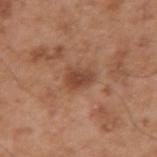Clinical impression: Part of a total-body skin-imaging series; this lesion was reviewed on a skin check and was not flagged for biopsy. Acquisition and patient details: A male patient in their mid- to late 50s. The lesion is located on the right upper arm. A 15 mm close-up extracted from a 3D total-body photography capture.